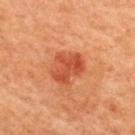Findings:
* notes · no biopsy performed (imaged during a skin exam)
* lighting · cross-polarized illumination
* TBP lesion metrics · an automated nevus-likeness rating near 85 out of 100 and lesion-presence confidence of about 100/100
* anatomic site · the back
* image source · ~15 mm crop, total-body skin-cancer survey
* subject · female, in their 40s